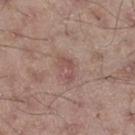| key | value |
|---|---|
| image | total-body-photography crop, ~15 mm field of view |
| illumination | white-light illumination |
| size | ≈3 mm |
| automated lesion analysis | a footprint of about 3.5 mm² and a symmetry-axis asymmetry near 0.55 |
| site | the leg |
| subject | male, aged 53–57 |